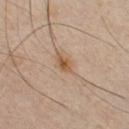Impression: Recorded during total-body skin imaging; not selected for excision or biopsy. Context: A male patient, aged approximately 35. An algorithmic analysis of the crop reported a lesion area of about 3 mm². The software also gave a lesion color around L≈51 a*≈18 b*≈32 in CIELAB, about 9 CIELAB-L* units darker than the surrounding skin, and a normalized lesion–skin contrast near 8. The software also gave lesion-presence confidence of about 100/100. The tile uses cross-polarized illumination. The recorded lesion diameter is about 2.5 mm. A 15 mm close-up tile from a total-body photography series done for melanoma screening. On the chest.